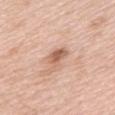Cropped from a whole-body photographic skin survey; the tile spans about 15 mm.
The patient is a female aged 63–67.
The lesion is on the upper back.